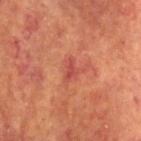Assessment: No biopsy was performed on this lesion — it was imaged during a full skin examination and was not determined to be concerning. Acquisition and patient details: A male subject approximately 55 years of age. The tile uses cross-polarized illumination. A 15 mm close-up tile from a total-body photography series done for melanoma screening. Located on the head or neck. Automated image analysis of the tile measured an automated nevus-likeness rating near 0 out of 100 and a detector confidence of about 100 out of 100 that the crop contains a lesion. Approximately 3 mm at its widest.